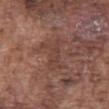This is a white-light tile.
This image is a 15 mm lesion crop taken from a total-body photograph.
A male patient about 75 years old.
The total-body-photography lesion software estimated a lesion area of about 8.5 mm², a shape eccentricity near 0.85, and two-axis asymmetry of about 0.5. The software also gave an average lesion color of about L≈41 a*≈20 b*≈24 (CIELAB), roughly 6 lightness units darker than nearby skin, and a normalized border contrast of about 5. The analysis additionally found a border-irregularity rating of about 7.5/10, a color-variation rating of about 2/10, and peripheral color asymmetry of about 0.5.
The lesion is located on the abdomen.